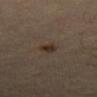Notes:
– follow-up — imaged on a skin check; not biopsied
– automated lesion analysis — a mean CIELAB color near L≈28 a*≈12 b*≈22 and a lesion–skin lightness drop of about 8; a detector confidence of about 100 out of 100 that the crop contains a lesion
– subject — male, aged 53–57
– lesion size — ≈2 mm
– location — the right thigh
– tile lighting — cross-polarized illumination
– acquisition — ~15 mm crop, total-body skin-cancer survey